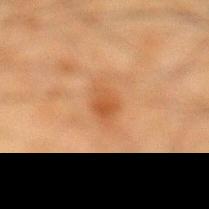automated lesion analysis: a lesion area of about 5 mm², an outline eccentricity of about 0.75 (0 = round, 1 = elongated), and a shape-asymmetry score of about 0.25 (0 = symmetric); acquisition: 15 mm crop, total-body photography; anatomic site: the left lower leg; subject: male, about 35 years old.